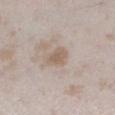Impression:
Imaged during a routine full-body skin examination; the lesion was not biopsied and no histopathology is available.
Clinical summary:
A female subject, roughly 25 years of age. The lesion is located on the leg. A close-up tile cropped from a whole-body skin photograph, about 15 mm across. Longest diameter approximately 3 mm. An algorithmic analysis of the crop reported roughly 8 lightness units darker than nearby skin and a lesion-to-skin contrast of about 6.5 (normalized; higher = more distinct). The analysis additionally found border irregularity of about 2 on a 0–10 scale and a within-lesion color-variation index near 1.5/10.